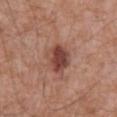{"biopsy_status": "not biopsied; imaged during a skin examination", "automated_metrics": {"eccentricity": 0.6, "cielab_L": 44, "cielab_a": 24, "cielab_b": 26}, "lesion_size": {"long_diameter_mm_approx": 3.5}, "site": "mid back", "patient": {"sex": "male", "age_approx": 60}, "lighting": "white-light", "image": {"source": "total-body photography crop", "field_of_view_mm": 15}}